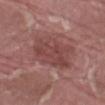follow-up: catalogued during a skin exam; not biopsied | size: about 6 mm | automated metrics: a lesion color around L≈44 a*≈24 b*≈22 in CIELAB and about 8 CIELAB-L* units darker than the surrounding skin; a border-irregularity rating of about 2.5/10, a color-variation rating of about 3.5/10, and a peripheral color-asymmetry measure near 1; an automated nevus-likeness rating near 0 out of 100 and lesion-presence confidence of about 100/100 | tile lighting: white-light illumination | imaging modality: ~15 mm tile from a whole-body skin photo | site: the left thigh | patient: male, aged approximately 40.